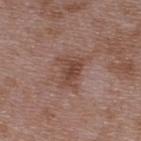biopsy status=catalogued during a skin exam; not biopsied | subject=male, aged approximately 50 | lesion diameter=≈4 mm | image-analysis metrics=internal color variation of about 3.5 on a 0–10 scale; an automated nevus-likeness rating near 0 out of 100 and a lesion-detection confidence of about 100/100 | lighting=white-light illumination | image source=15 mm crop, total-body photography | location=the upper back.